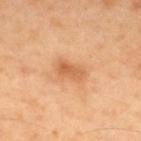<tbp_lesion>
  <biopsy_status>not biopsied; imaged during a skin examination</biopsy_status>
  <lighting>cross-polarized</lighting>
  <image>
    <source>total-body photography crop</source>
    <field_of_view_mm>15</field_of_view_mm>
  </image>
  <patient>
    <sex>male</sex>
    <age_approx>40</age_approx>
  </patient>
  <site>upper back</site>
  <automated_metrics>
    <cielab_L>62</cielab_L>
    <cielab_a>25</cielab_a>
    <cielab_b>41</cielab_b>
    <vs_skin_darker_L>10.0</vs_skin_darker_L>
    <vs_skin_contrast_norm>6.5</vs_skin_contrast_norm>
    <lesion_detection_confidence_0_100>100</lesion_detection_confidence_0_100>
  </automated_metrics>
  <lesion_size>
    <long_diameter_mm_approx>3.5</long_diameter_mm_approx>
  </lesion_size>
</tbp_lesion>